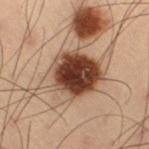* workup: no biopsy performed (imaged during a skin exam)
* anatomic site: the right thigh
* imaging modality: ~15 mm tile from a whole-body skin photo
* patient: male, in their mid-50s
* TBP lesion metrics: a footprint of about 24 mm², an outline eccentricity of about 0.7 (0 = round, 1 = elongated), and a shape-asymmetry score of about 0.2 (0 = symmetric); a lesion color around L≈35 a*≈17 b*≈24 in CIELAB, a lesion–skin lightness drop of about 17, and a normalized border contrast of about 14; an automated nevus-likeness rating near 100 out of 100 and a lesion-detection confidence of about 100/100